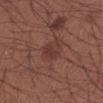Impression:
Imaged during a routine full-body skin examination; the lesion was not biopsied and no histopathology is available.